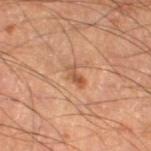Imaged during a routine full-body skin examination; the lesion was not biopsied and no histopathology is available. A male subject, aged around 60. Automated tile analysis of the lesion measured an area of roughly 3.5 mm², an eccentricity of roughly 0.85, and a symmetry-axis asymmetry near 0.4. And it measured a mean CIELAB color near L≈50 a*≈22 b*≈32, a lesion–skin lightness drop of about 9, and a normalized border contrast of about 6.5. On the left thigh. A roughly 15 mm field-of-view crop from a total-body skin photograph. Longest diameter approximately 3 mm. Captured under cross-polarized illumination.